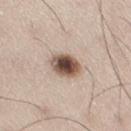Part of a total-body skin-imaging series; this lesion was reviewed on a skin check and was not flagged for biopsy.
The lesion is located on the right thigh.
A 15 mm close-up tile from a total-body photography series done for melanoma screening.
Approximately 3.5 mm at its widest.
A male patient, in their 40s.